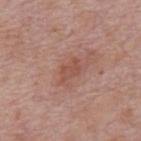The lesion was tiled from a total-body skin photograph and was not biopsied. The lesion is located on the back. The recorded lesion diameter is about 3 mm. A 15 mm crop from a total-body photograph taken for skin-cancer surveillance. Automated image analysis of the tile measured an eccentricity of roughly 0.8. The software also gave an average lesion color of about L≈51 a*≈23 b*≈27 (CIELAB), roughly 7 lightness units darker than nearby skin, and a lesion-to-skin contrast of about 5.5 (normalized; higher = more distinct). The software also gave an automated nevus-likeness rating near 5 out of 100. The subject is a male roughly 75 years of age. Imaged with white-light lighting.